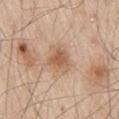Q: Was this lesion biopsied?
A: catalogued during a skin exam; not biopsied
Q: Lesion location?
A: the right thigh
Q: Who is the patient?
A: male, in their 60s
Q: What is the lesion's diameter?
A: ≈3 mm
Q: How was the tile lit?
A: white-light
Q: What kind of image is this?
A: 15 mm crop, total-body photography